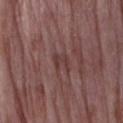Q: Was this lesion biopsied?
A: catalogued during a skin exam; not biopsied
Q: What kind of image is this?
A: total-body-photography crop, ~15 mm field of view
Q: Who is the patient?
A: female, aged 68 to 72
Q: What is the anatomic site?
A: the right upper arm
Q: Illumination type?
A: white-light
Q: What is the lesion's diameter?
A: about 2.5 mm